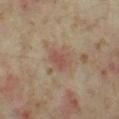biopsy status: total-body-photography surveillance lesion; no biopsy
lesion size: ~3 mm (longest diameter)
tile lighting: cross-polarized
image source: ~15 mm crop, total-body skin-cancer survey
patient: female, roughly 35 years of age
location: the left thigh